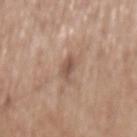Clinical impression: This lesion was catalogued during total-body skin photography and was not selected for biopsy. Context: This is a white-light tile. Automated tile analysis of the lesion measured a footprint of about 4 mm², an outline eccentricity of about 0.85 (0 = round, 1 = elongated), and two-axis asymmetry of about 0.3. The software also gave a border-irregularity rating of about 3/10, a color-variation rating of about 2/10, and peripheral color asymmetry of about 0.5. The analysis additionally found an automated nevus-likeness rating near 5 out of 100 and a detector confidence of about 100 out of 100 that the crop contains a lesion. A roughly 15 mm field-of-view crop from a total-body skin photograph. The lesion's longest dimension is about 3 mm. The lesion is on the back. A male subject, aged 68–72.